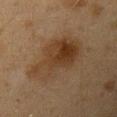notes: total-body-photography surveillance lesion; no biopsy | size: ~8 mm (longest diameter) | subject: female, roughly 40 years of age | body site: the left upper arm | imaging modality: ~15 mm crop, total-body skin-cancer survey.